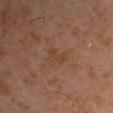  biopsy_status: not biopsied; imaged during a skin examination
  lighting: cross-polarized
  lesion_size:
    long_diameter_mm_approx: 3.0
  image:
    source: total-body photography crop
    field_of_view_mm: 15
  automated_metrics:
    area_mm2_approx: 4.5
    eccentricity: 0.8
    shape_asymmetry: 0.4
    border_irregularity_0_10: 4.5
    color_variation_0_10: 1.5
    peripheral_color_asymmetry: 0.5
  patient:
    sex: male
    age_approx: 60
  site: left upper arm